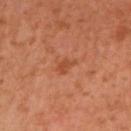<lesion>
  <biopsy_status>not biopsied; imaged during a skin examination</biopsy_status>
  <lesion_size>
    <long_diameter_mm_approx>2.5</long_diameter_mm_approx>
  </lesion_size>
  <lighting>cross-polarized</lighting>
  <image>
    <source>total-body photography crop</source>
    <field_of_view_mm>15</field_of_view_mm>
  </image>
  <patient>
    <sex>female</sex>
    <age_approx>40</age_approx>
  </patient>
  <site>arm</site>
</lesion>